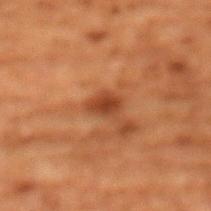follow-up — total-body-photography surveillance lesion; no biopsy | location — the chest | lighting — cross-polarized | imaging modality — ~15 mm crop, total-body skin-cancer survey | diameter — ~2.5 mm (longest diameter) | patient — female, in their 80s.